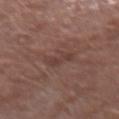The lesion was photographed on a routine skin check and not biopsied; there is no pathology result.
Measured at roughly 4 mm in maximum diameter.
A 15 mm crop from a total-body photograph taken for skin-cancer surveillance.
The total-body-photography lesion software estimated a mean CIELAB color near L≈39 a*≈19 b*≈22, roughly 6 lightness units darker than nearby skin, and a lesion-to-skin contrast of about 5.5 (normalized; higher = more distinct). The software also gave radial color variation of about 0.
A male patient, aged approximately 55.
This is a white-light tile.
From the right forearm.